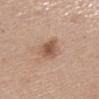workup: total-body-photography surveillance lesion; no biopsy | tile lighting: white-light | anatomic site: the back | patient: female, aged 58–62 | automated metrics: a footprint of about 6.5 mm², an eccentricity of roughly 0.9, and two-axis asymmetry of about 0.2; an average lesion color of about L≈54 a*≈19 b*≈29 (CIELAB), roughly 11 lightness units darker than nearby skin, and a normalized lesion–skin contrast near 8; a border-irregularity index near 3/10, a color-variation rating of about 4/10, and a peripheral color-asymmetry measure near 1 | imaging modality: 15 mm crop, total-body photography.